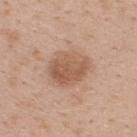{"biopsy_status": "not biopsied; imaged during a skin examination", "automated_metrics": {"area_mm2_approx": 15.0, "shape_asymmetry": 0.15, "cielab_L": 57, "cielab_a": 20, "cielab_b": 30, "vs_skin_darker_L": 10.0, "vs_skin_contrast_norm": 7.0, "border_irregularity_0_10": 1.5, "peripheral_color_asymmetry": 1.5}, "patient": {"sex": "female", "age_approx": 40}, "image": {"source": "total-body photography crop", "field_of_view_mm": 15}, "site": "back", "lighting": "white-light"}